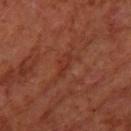Notes:
– notes · catalogued during a skin exam; not biopsied
– acquisition · ~15 mm crop, total-body skin-cancer survey
– image-analysis metrics · internal color variation of about 0 on a 0–10 scale and a peripheral color-asymmetry measure near 0; a classifier nevus-likeness of about 0/100 and lesion-presence confidence of about 95/100
– subject · female, roughly 65 years of age
– anatomic site · the left forearm